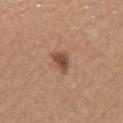– biopsy status — imaged on a skin check; not biopsied
– subject — female, approximately 60 years of age
– lesion diameter — ≈2.5 mm
– site — the left forearm
– automated metrics — a border-irregularity rating of about 3/10 and internal color variation of about 2.5 on a 0–10 scale
– imaging modality — total-body-photography crop, ~15 mm field of view
– illumination — white-light